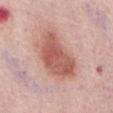Q: Is there a histopathology result?
A: total-body-photography surveillance lesion; no biopsy
Q: Where on the body is the lesion?
A: the abdomen
Q: How large is the lesion?
A: ~7 mm (longest diameter)
Q: What are the patient's age and sex?
A: female, roughly 65 years of age
Q: Automated lesion metrics?
A: roughly 13 lightness units darker than nearby skin; a border-irregularity rating of about 3/10; an automated nevus-likeness rating near 100 out of 100 and lesion-presence confidence of about 100/100
Q: What is the imaging modality?
A: 15 mm crop, total-body photography